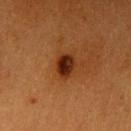Assessment:
Part of a total-body skin-imaging series; this lesion was reviewed on a skin check and was not flagged for biopsy.
Background:
A lesion tile, about 15 mm wide, cut from a 3D total-body photograph. The lesion-visualizer software estimated an eccentricity of roughly 0.6 and a shape-asymmetry score of about 0.2 (0 = symmetric). The software also gave an average lesion color of about L≈24 a*≈23 b*≈29 (CIELAB), roughly 13 lightness units darker than nearby skin, and a normalized border contrast of about 13. The lesion is on the left upper arm. A female subject roughly 55 years of age.